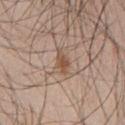Impression: No biopsy was performed on this lesion — it was imaged during a full skin examination and was not determined to be concerning. Acquisition and patient details: Located on the chest. The recorded lesion diameter is about 3 mm. Cropped from a total-body skin-imaging series; the visible field is about 15 mm. Automated image analysis of the tile measured a footprint of about 4 mm² and a shape-asymmetry score of about 0.3 (0 = symmetric). It also reported a peripheral color-asymmetry measure near 0.5. The tile uses white-light illumination. A male patient, approximately 45 years of age.